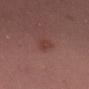This lesion was catalogued during total-body skin photography and was not selected for biopsy.
The lesion is located on the left thigh.
Longest diameter approximately 3 mm.
The subject is a male aged around 40.
Captured under white-light illumination.
A 15 mm close-up extracted from a 3D total-body photography capture.